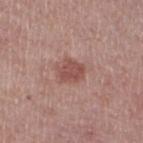biopsy status: imaged on a skin check; not biopsied
patient: male, aged 73–77
automated lesion analysis: a lesion area of about 6 mm², a shape eccentricity near 0.55, and a shape-asymmetry score of about 0.25 (0 = symmetric); roughly 10 lightness units darker than nearby skin and a lesion-to-skin contrast of about 7.5 (normalized; higher = more distinct)
image: 15 mm crop, total-body photography
lesion size: ≈3 mm
location: the right thigh